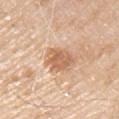The lesion was photographed on a routine skin check and not biopsied; there is no pathology result. A male subject aged approximately 80. Imaged with white-light lighting. A lesion tile, about 15 mm wide, cut from a 3D total-body photograph. On the arm.